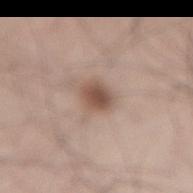The lesion was photographed on a routine skin check and not biopsied; there is no pathology result. Measured at roughly 3 mm in maximum diameter. A male patient approximately 35 years of age. Automated image analysis of the tile measured an area of roughly 5.5 mm², an eccentricity of roughly 0.6, and a shape-asymmetry score of about 0.2 (0 = symmetric). The analysis additionally found a lesion color around L≈52 a*≈18 b*≈26 in CIELAB, a lesion–skin lightness drop of about 12, and a normalized lesion–skin contrast near 8.5. It also reported a nevus-likeness score of about 90/100 and a detector confidence of about 100 out of 100 that the crop contains a lesion. This is a white-light tile. Located on the lower back. Cropped from a whole-body photographic skin survey; the tile spans about 15 mm.